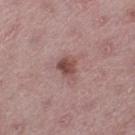Findings:
– workup — total-body-photography surveillance lesion; no biopsy
– body site — the left thigh
– lighting — white-light illumination
– size — ≈3 mm
– automated metrics — a lesion area of about 4.5 mm² and an eccentricity of roughly 0.6; internal color variation of about 4 on a 0–10 scale and peripheral color asymmetry of about 1.5; a nevus-likeness score of about 80/100
– image — ~15 mm tile from a whole-body skin photo
– patient — female, in their mid-40s